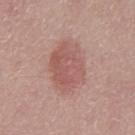follow-up: imaged on a skin check; not biopsied | lighting: white-light illumination | body site: the abdomen | subject: male, in their mid-40s | lesion size: ~6 mm (longest diameter) | acquisition: 15 mm crop, total-body photography.